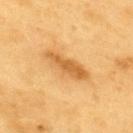biopsy status = imaged on a skin check; not biopsied | anatomic site = the upper back | patient = male, aged around 60 | image source = ~15 mm tile from a whole-body skin photo.